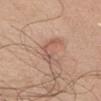Q: Was a biopsy performed?
A: catalogued during a skin exam; not biopsied
Q: What is the lesion's diameter?
A: ≈5 mm
Q: How was the tile lit?
A: white-light
Q: What are the patient's age and sex?
A: male, in their mid- to late 50s
Q: What is the imaging modality?
A: ~15 mm tile from a whole-body skin photo
Q: Lesion location?
A: the chest